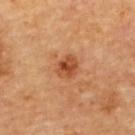Captured during whole-body skin photography for melanoma surveillance; the lesion was not biopsied. This is a cross-polarized tile. The recorded lesion diameter is about 2.5 mm. Located on the upper back. Automated image analysis of the tile measured an area of roughly 5 mm² and an outline eccentricity of about 0.55 (0 = round, 1 = elongated). It also reported internal color variation of about 5 on a 0–10 scale and a peripheral color-asymmetry measure near 2. Cropped from a whole-body photographic skin survey; the tile spans about 15 mm. A male patient in their mid-80s or older.